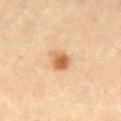Case summary:
* follow-up · catalogued during a skin exam; not biopsied
* image · ~15 mm tile from a whole-body skin photo
* automated lesion analysis · a lesion color around L≈55 a*≈20 b*≈35 in CIELAB and roughly 12 lightness units darker than nearby skin; a color-variation rating of about 4.5/10 and peripheral color asymmetry of about 1.5
* tile lighting · cross-polarized illumination
* anatomic site · the right thigh
* patient · female, in their mid- to late 40s
* size · ~2.5 mm (longest diameter)